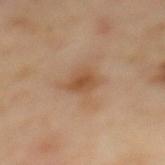Impression:
The lesion was tiled from a total-body skin photograph and was not biopsied.
Image and clinical context:
A female patient aged approximately 60. This is a cross-polarized tile. The lesion is on the back. Measured at roughly 2.5 mm in maximum diameter. A close-up tile cropped from a whole-body skin photograph, about 15 mm across.